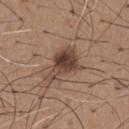The lesion was tiled from a total-body skin photograph and was not biopsied. The lesion-visualizer software estimated a mean CIELAB color near L≈43 a*≈17 b*≈25, roughly 13 lightness units darker than nearby skin, and a normalized border contrast of about 10. The analysis additionally found an automated nevus-likeness rating near 90 out of 100. Imaged with white-light lighting. A male patient, aged around 65. The recorded lesion diameter is about 4.5 mm. On the chest. A close-up tile cropped from a whole-body skin photograph, about 15 mm across.